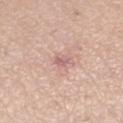biopsy status: no biopsy performed (imaged during a skin exam)
image source: total-body-photography crop, ~15 mm field of view
patient: female, aged 43 to 47
lighting: white-light
site: the left lower leg
size: ~2 mm (longest diameter)
automated lesion analysis: a lesion area of about 2 mm², a shape eccentricity near 0.65, and a symmetry-axis asymmetry near 0.3; about 9 CIELAB-L* units darker than the surrounding skin